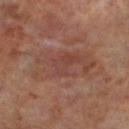Clinical impression:
Part of a total-body skin-imaging series; this lesion was reviewed on a skin check and was not flagged for biopsy.
Clinical summary:
A male patient, aged 68–72. On the left lower leg. Imaged with cross-polarized lighting. A region of skin cropped from a whole-body photographic capture, roughly 15 mm wide. The recorded lesion diameter is about 8 mm.